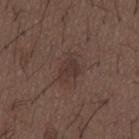The lesion is on the mid back. Automated image analysis of the tile measured a lesion area of about 5 mm², a shape eccentricity near 0.65, and a shape-asymmetry score of about 0.3 (0 = symmetric). And it measured a border-irregularity index near 3/10. It also reported a detector confidence of about 100 out of 100 that the crop contains a lesion. The patient is a male in their 50s. A 15 mm close-up extracted from a 3D total-body photography capture. The recorded lesion diameter is about 3 mm.